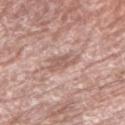{"biopsy_status": "not biopsied; imaged during a skin examination", "automated_metrics": {"eccentricity": 0.85, "shape_asymmetry": 0.3, "cielab_L": 58, "cielab_a": 20, "cielab_b": 25, "vs_skin_darker_L": 9.0, "vs_skin_contrast_norm": 6.0, "nevus_likeness_0_100": 0, "lesion_detection_confidence_0_100": 75}, "site": "right upper arm", "lesion_size": {"long_diameter_mm_approx": 3.5}, "patient": {"sex": "male", "age_approx": 70}, "image": {"source": "total-body photography crop", "field_of_view_mm": 15}, "lighting": "white-light"}